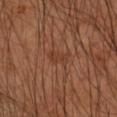  biopsy_status: not biopsied; imaged during a skin examination
  lesion_size:
    long_diameter_mm_approx: 2.5
  site: right forearm
  image:
    source: total-body photography crop
    field_of_view_mm: 15
  patient:
    sex: male
    age_approx: 50